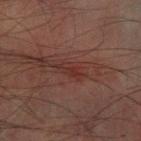Captured during whole-body skin photography for melanoma surveillance; the lesion was not biopsied.